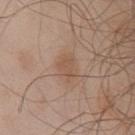Case summary:
– workup — catalogued during a skin exam; not biopsied
– lesion size — about 3.5 mm
– illumination — white-light illumination
– body site — the chest
– automated metrics — a footprint of about 5 mm², an eccentricity of roughly 0.8, and a symmetry-axis asymmetry near 0.35
– subject — male, about 55 years old
– imaging modality — ~15 mm crop, total-body skin-cancer survey